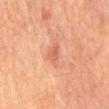anatomic site: the mid back | patient: male, approximately 75 years of age | imaging modality: total-body-photography crop, ~15 mm field of view.